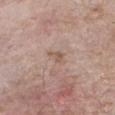workup: imaged on a skin check; not biopsied
image source: ~15 mm crop, total-body skin-cancer survey
subject: male, approximately 60 years of age
automated lesion analysis: an outline eccentricity of about 0.85 (0 = round, 1 = elongated) and a shape-asymmetry score of about 0.55 (0 = symmetric); an automated nevus-likeness rating near 0 out of 100 and lesion-presence confidence of about 100/100
size: ≈3 mm
body site: the arm
tile lighting: white-light illumination